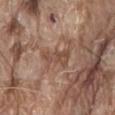workup: catalogued during a skin exam; not biopsied | image: total-body-photography crop, ~15 mm field of view | location: the front of the torso | illumination: white-light | patient: male, roughly 80 years of age | image-analysis metrics: a mean CIELAB color near L≈48 a*≈19 b*≈27, roughly 7 lightness units darker than nearby skin, and a normalized border contrast of about 6.